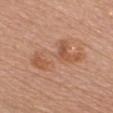follow-up = imaged on a skin check; not biopsied
imaging modality = ~15 mm crop, total-body skin-cancer survey
subject = female, aged around 60
site = the chest